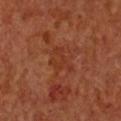Impression:
Part of a total-body skin-imaging series; this lesion was reviewed on a skin check and was not flagged for biopsy.
Context:
The lesion is located on the chest. The subject is a female aged approximately 65. Captured under cross-polarized illumination. About 3.5 mm across. A region of skin cropped from a whole-body photographic capture, roughly 15 mm wide.